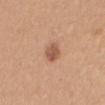Impression:
The lesion was photographed on a routine skin check and not biopsied; there is no pathology result.
Clinical summary:
Captured under white-light illumination. The lesion-visualizer software estimated a mean CIELAB color near L≈55 a*≈23 b*≈32 and roughly 11 lightness units darker than nearby skin. And it measured border irregularity of about 2 on a 0–10 scale and peripheral color asymmetry of about 1. And it measured a lesion-detection confidence of about 100/100. Located on the back. The lesion's longest dimension is about 3 mm. A 15 mm crop from a total-body photograph taken for skin-cancer surveillance. The patient is a female in their mid- to late 50s.